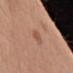The patient is a male in their mid-50s.
A close-up tile cropped from a whole-body skin photograph, about 15 mm across.
The recorded lesion diameter is about 2.5 mm.
From the left upper arm.
The tile uses white-light illumination.
The lesion-visualizer software estimated a lesion area of about 2.5 mm². It also reported a color-variation rating of about 0.5/10 and peripheral color asymmetry of about 0.